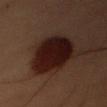The lesion is on the right forearm. This is a cross-polarized tile. A male subject aged 53–57. A region of skin cropped from a whole-body photographic capture, roughly 15 mm wide. Measured at roughly 7 mm in maximum diameter.